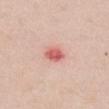The lesion was photographed on a routine skin check and not biopsied; there is no pathology result. Located on the chest. A female patient, aged around 50. A 15 mm close-up tile from a total-body photography series done for melanoma screening. The lesion-visualizer software estimated a lesion area of about 4.5 mm², an eccentricity of roughly 0.6, and a shape-asymmetry score of about 0.2 (0 = symmetric). The analysis additionally found a nevus-likeness score of about 0/100 and a lesion-detection confidence of about 100/100. Measured at roughly 2.5 mm in maximum diameter.